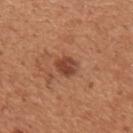notes=total-body-photography surveillance lesion; no biopsy | diameter=about 2.5 mm | patient=male, aged approximately 55 | TBP lesion metrics=an area of roughly 5 mm²; a classifier nevus-likeness of about 80/100 and a detector confidence of about 100 out of 100 that the crop contains a lesion | image source=~15 mm crop, total-body skin-cancer survey | body site=the upper back | lighting=white-light.